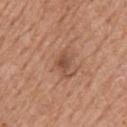image source: ~15 mm crop, total-body skin-cancer survey; subject: male, aged 58 to 62; body site: the back.